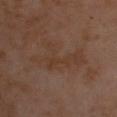No biopsy was performed on this lesion — it was imaged during a full skin examination and was not determined to be concerning.
Located on the chest.
This is a cross-polarized tile.
A male patient, in their mid-50s.
Automated image analysis of the tile measured roughly 4 lightness units darker than nearby skin. The software also gave a classifier nevus-likeness of about 0/100 and a lesion-detection confidence of about 95/100.
A 15 mm close-up tile from a total-body photography series done for melanoma screening.